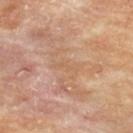| key | value |
|---|---|
| notes | no biopsy performed (imaged during a skin exam) |
| patient | male, about 85 years old or older |
| tile lighting | cross-polarized |
| body site | the upper back |
| acquisition | 15 mm crop, total-body photography |